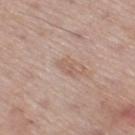Case summary:
– follow-up — no biopsy performed (imaged during a skin exam)
– image — 15 mm crop, total-body photography
– subject — male, aged 68–72
– body site — the right thigh